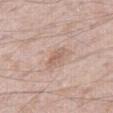{"site": "right thigh", "automated_metrics": {"area_mm2_approx": 3.0, "shape_asymmetry": 0.2, "cielab_L": 60, "cielab_a": 18, "cielab_b": 27, "nevus_likeness_0_100": 10, "lesion_detection_confidence_0_100": 100}, "patient": {"sex": "male", "age_approx": 65}, "image": {"source": "total-body photography crop", "field_of_view_mm": 15}, "lighting": "white-light"}